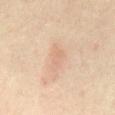Part of a total-body skin-imaging series; this lesion was reviewed on a skin check and was not flagged for biopsy.
The lesion is located on the abdomen.
The total-body-photography lesion software estimated an area of roughly 5.5 mm². The software also gave a border-irregularity rating of about 3.5/10, a within-lesion color-variation index near 1.5/10, and peripheral color asymmetry of about 0.5. And it measured a detector confidence of about 100 out of 100 that the crop contains a lesion.
Cropped from a total-body skin-imaging series; the visible field is about 15 mm.
The subject is a male roughly 65 years of age.
Approximately 3.5 mm at its widest.